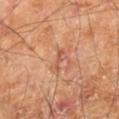Findings:
* workup · catalogued during a skin exam; not biopsied
* size · about 3 mm
* automated lesion analysis · a lesion area of about 3 mm², a shape eccentricity near 0.9, and a shape-asymmetry score of about 0.45 (0 = symmetric); a border-irregularity index near 5/10, internal color variation of about 2 on a 0–10 scale, and a peripheral color-asymmetry measure near 0.5; a classifier nevus-likeness of about 0/100 and a lesion-detection confidence of about 100/100
* patient · male, approximately 70 years of age
* imaging modality · ~15 mm crop, total-body skin-cancer survey
* body site · the right lower leg